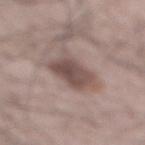This lesion was catalogued during total-body skin photography and was not selected for biopsy. The lesion's longest dimension is about 6 mm. Cropped from a total-body skin-imaging series; the visible field is about 15 mm. From the back. Captured under white-light illumination. A male patient in their mid- to late 60s.